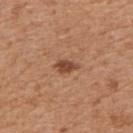Acquisition and patient details:
A male patient in their mid- to late 50s. A region of skin cropped from a whole-body photographic capture, roughly 15 mm wide. Located on the right upper arm.